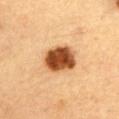Case summary:
- workup · catalogued during a skin exam; not biopsied
- patient · male, aged around 85 or older
- image source · ~15 mm tile from a whole-body skin photo
- lighting · cross-polarized illumination
- anatomic site · the abdomen